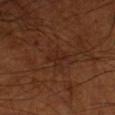Longest diameter approximately 2.5 mm.
A male subject in their 70s.
Imaged with cross-polarized lighting.
The total-body-photography lesion software estimated a mean CIELAB color near L≈24 a*≈20 b*≈25 and a lesion–skin lightness drop of about 4. The analysis additionally found a border-irregularity index near 2/10, internal color variation of about 2 on a 0–10 scale, and radial color variation of about 0.5. The software also gave a detector confidence of about 100 out of 100 that the crop contains a lesion.
A roughly 15 mm field-of-view crop from a total-body skin photograph.
The lesion is on the left forearm.